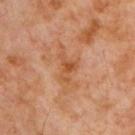Q: What are the patient's age and sex?
A: male, aged approximately 60
Q: Automated lesion metrics?
A: a footprint of about 5 mm², a shape eccentricity near 0.8, and a symmetry-axis asymmetry near 0.6; a mean CIELAB color near L≈49 a*≈24 b*≈36 and roughly 7 lightness units darker than nearby skin; a border-irregularity rating of about 6.5/10, a color-variation rating of about 3/10, and radial color variation of about 0.5; an automated nevus-likeness rating near 0 out of 100 and a lesion-detection confidence of about 100/100
Q: How was this image acquired?
A: total-body-photography crop, ~15 mm field of view
Q: Where on the body is the lesion?
A: the chest